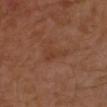| key | value |
|---|---|
| notes | total-body-photography surveillance lesion; no biopsy |
| lesion diameter | ≈3.5 mm |
| subject | male, roughly 30 years of age |
| location | the left forearm |
| tile lighting | cross-polarized illumination |
| acquisition | 15 mm crop, total-body photography |
| automated lesion analysis | an average lesion color of about L≈37 a*≈21 b*≈29 (CIELAB), a lesion–skin lightness drop of about 5, and a normalized border contrast of about 5; a color-variation rating of about 2/10 and peripheral color asymmetry of about 0.5 |